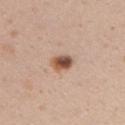Impression:
Imaged during a routine full-body skin examination; the lesion was not biopsied and no histopathology is available.
Image and clinical context:
From the arm. A female subject in their 20s. This is a white-light tile. Cropped from a whole-body photographic skin survey; the tile spans about 15 mm.